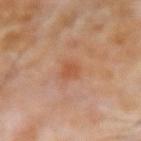Impression:
Part of a total-body skin-imaging series; this lesion was reviewed on a skin check and was not flagged for biopsy.
Background:
The lesion-visualizer software estimated a border-irregularity rating of about 2/10, a within-lesion color-variation index near 1.5/10, and peripheral color asymmetry of about 0.5. And it measured a classifier nevus-likeness of about 15/100. The tile uses cross-polarized illumination. About 2.5 mm across. The lesion is located on the right forearm. The patient is a male roughly 70 years of age. A lesion tile, about 15 mm wide, cut from a 3D total-body photograph.